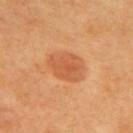The lesion was photographed on a routine skin check and not biopsied; there is no pathology result. On the back. A female patient, roughly 60 years of age. A lesion tile, about 15 mm wide, cut from a 3D total-body photograph.